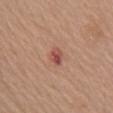Q: Was a biopsy performed?
A: catalogued during a skin exam; not biopsied
Q: Lesion size?
A: about 3.5 mm
Q: What is the anatomic site?
A: the upper back
Q: Automated lesion metrics?
A: a detector confidence of about 100 out of 100 that the crop contains a lesion
Q: Who is the patient?
A: female, roughly 70 years of age
Q: How was this image acquired?
A: ~15 mm tile from a whole-body skin photo